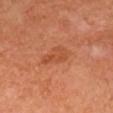Assessment: Imaged during a routine full-body skin examination; the lesion was not biopsied and no histopathology is available. Image and clinical context: The lesion's longest dimension is about 3.5 mm. Automated image analysis of the tile measured a lesion area of about 6 mm², an eccentricity of roughly 0.8, and a shape-asymmetry score of about 0.4 (0 = symmetric). A female patient roughly 55 years of age. A 15 mm crop from a total-body photograph taken for skin-cancer surveillance. Located on the head or neck.